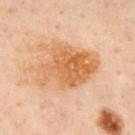Q: Was a biopsy performed?
A: imaged on a skin check; not biopsied
Q: What is the imaging modality?
A: 15 mm crop, total-body photography
Q: Where on the body is the lesion?
A: the back
Q: Who is the patient?
A: male, aged 48–52
Q: What did automated image analysis measure?
A: a lesion area of about 27 mm², an outline eccentricity of about 0.8 (0 = round, 1 = elongated), and a shape-asymmetry score of about 0.25 (0 = symmetric); a nevus-likeness score of about 20/100
Q: Illumination type?
A: cross-polarized illumination